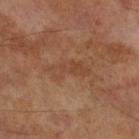Recorded during total-body skin imaging; not selected for excision or biopsy.
On the right lower leg.
Captured under cross-polarized illumination.
This image is a 15 mm lesion crop taken from a total-body photograph.
A male subject aged 68 to 72.
Approximately 5 mm at its widest.